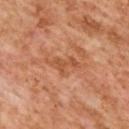Part of a total-body skin-imaging series; this lesion was reviewed on a skin check and was not flagged for biopsy.
This image is a 15 mm lesion crop taken from a total-body photograph.
A female patient in their 60s.
From the upper back.
Approximately 4 mm at its widest.
Imaged with cross-polarized lighting.
Automated image analysis of the tile measured an area of roughly 5.5 mm², an eccentricity of roughly 0.85, and two-axis asymmetry of about 0.55.